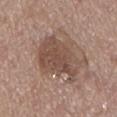  biopsy_status: not biopsied; imaged during a skin examination
  patient:
    sex: male
    age_approx: 70
  automated_metrics:
    area_mm2_approx: 24.0
    eccentricity: 0.15
    shape_asymmetry: 0.25
    cielab_L: 49
    cielab_a: 17
    cielab_b: 25
    vs_skin_darker_L: 10.0
    vs_skin_contrast_norm: 7.0
    border_irregularity_0_10: 4.0
    color_variation_0_10: 3.5
    peripheral_color_asymmetry: 1.0
    nevus_likeness_0_100: 10
  image:
    source: total-body photography crop
    field_of_view_mm: 15
  lighting: white-light
  lesion_size:
    long_diameter_mm_approx: 6.0
  site: back